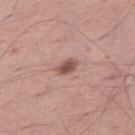Clinical impression:
No biopsy was performed on this lesion — it was imaged during a full skin examination and was not determined to be concerning.
Context:
A male subject, aged around 60. The tile uses white-light illumination. Measured at roughly 3 mm in maximum diameter. The lesion is located on the leg. Cropped from a whole-body photographic skin survey; the tile spans about 15 mm.